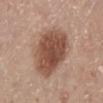{
  "biopsy_status": "not biopsied; imaged during a skin examination"
}